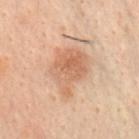Assessment: This lesion was catalogued during total-body skin photography and was not selected for biopsy. Context: The lesion's longest dimension is about 5.5 mm. A male patient approximately 50 years of age. A lesion tile, about 15 mm wide, cut from a 3D total-body photograph. The tile uses cross-polarized illumination. The lesion is located on the chest.